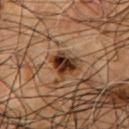Imaged during a routine full-body skin examination; the lesion was not biopsied and no histopathology is available. On the front of the torso. A roughly 15 mm field-of-view crop from a total-body skin photograph. A male subject about 55 years old. Captured under cross-polarized illumination.